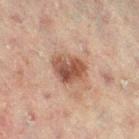notes — total-body-photography surveillance lesion; no biopsy
image source — total-body-photography crop, ~15 mm field of view
subject — male, aged around 45
location — the right thigh
size — ~4 mm (longest diameter)
lighting — cross-polarized illumination